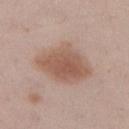Findings:
– biopsy status · no biopsy performed (imaged during a skin exam)
– acquisition · ~15 mm crop, total-body skin-cancer survey
– patient · female, aged approximately 20
– lesion diameter · about 7 mm
– anatomic site · the right lower leg
– lighting · white-light